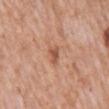Findings:
– follow-up · total-body-photography surveillance lesion; no biopsy
– subject · male, aged 53 to 57
– acquisition · ~15 mm crop, total-body skin-cancer survey
– diameter · ~2.5 mm (longest diameter)
– anatomic site · the mid back